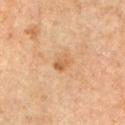Q: Was a biopsy performed?
A: total-body-photography surveillance lesion; no biopsy
Q: What lighting was used for the tile?
A: cross-polarized
Q: Who is the patient?
A: male, aged approximately 65
Q: What did automated image analysis measure?
A: an area of roughly 4 mm² and an outline eccentricity of about 0.7 (0 = round, 1 = elongated); an average lesion color of about L≈52 a*≈19 b*≈34 (CIELAB) and a lesion–skin lightness drop of about 7; an automated nevus-likeness rating near 5 out of 100
Q: Lesion location?
A: the arm
Q: What kind of image is this?
A: 15 mm crop, total-body photography
Q: Lesion size?
A: ~2.5 mm (longest diameter)